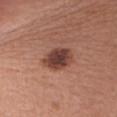Clinical impression:
Part of a total-body skin-imaging series; this lesion was reviewed on a skin check and was not flagged for biopsy.
Background:
The lesion-visualizer software estimated an automated nevus-likeness rating near 70 out of 100 and lesion-presence confidence of about 100/100. The subject is a female aged 53–57. The lesion is on the head or neck. Imaged with white-light lighting. Cropped from a whole-body photographic skin survey; the tile spans about 15 mm.